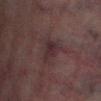biopsy status — imaged on a skin check; not biopsied
image — total-body-photography crop, ~15 mm field of view
illumination — cross-polarized illumination
patient — male, roughly 75 years of age
size — ~3.5 mm (longest diameter)
body site — the right lower leg